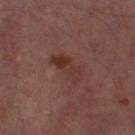workup=no biopsy performed (imaged during a skin exam) | subject=male, in their mid- to late 60s | acquisition=total-body-photography crop, ~15 mm field of view | illumination=cross-polarized | location=the left thigh.